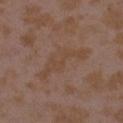biopsy status = no biopsy performed (imaged during a skin exam); anatomic site = the left upper arm; subject = female, aged 33 to 37; illumination = white-light; imaging modality = 15 mm crop, total-body photography; lesion size = about 7 mm.